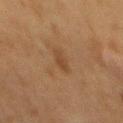The lesion is located on the mid back.
Approximately 3 mm at its widest.
A male subject in their mid-70s.
A 15 mm close-up tile from a total-body photography series done for melanoma screening.
The lesion-visualizer software estimated a footprint of about 3 mm² and an outline eccentricity of about 0.9 (0 = round, 1 = elongated). It also reported an average lesion color of about L≈35 a*≈16 b*≈28 (CIELAB), about 6 CIELAB-L* units darker than the surrounding skin, and a lesion-to-skin contrast of about 6 (normalized; higher = more distinct). The software also gave border irregularity of about 4 on a 0–10 scale, a within-lesion color-variation index near 0/10, and a peripheral color-asymmetry measure near 0. The software also gave an automated nevus-likeness rating near 5 out of 100 and a detector confidence of about 100 out of 100 that the crop contains a lesion.
Captured under cross-polarized illumination.